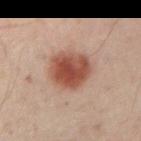Assessment: The lesion was photographed on a routine skin check and not biopsied; there is no pathology result. Background: The subject is a male about 50 years old. Cropped from a total-body skin-imaging series; the visible field is about 15 mm. The lesion is on the right upper arm. The tile uses cross-polarized illumination.